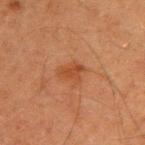follow-up: catalogued during a skin exam; not biopsied | lighting: cross-polarized illumination | TBP lesion metrics: a nevus-likeness score of about 20/100 and a detector confidence of about 100 out of 100 that the crop contains a lesion | patient: male, aged 43–47 | size: about 2.5 mm | acquisition: total-body-photography crop, ~15 mm field of view | site: the upper back.